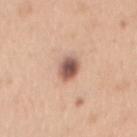<case>
<biopsy_status>not biopsied; imaged during a skin examination</biopsy_status>
<lighting>white-light</lighting>
<image>
  <source>total-body photography crop</source>
  <field_of_view_mm>15</field_of_view_mm>
</image>
<lesion_size>
  <long_diameter_mm_approx>3.5</long_diameter_mm_approx>
</lesion_size>
<site>chest</site>
<patient>
  <sex>female</sex>
  <age_approx>30</age_approx>
</patient>
</case>